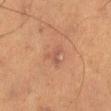Impression:
Recorded during total-body skin imaging; not selected for excision or biopsy.
Image and clinical context:
Imaged with cross-polarized lighting. The lesion is located on the leg. Longest diameter approximately 3 mm. Automated image analysis of the tile measured a border-irregularity index near 5/10 and a within-lesion color-variation index near 2.5/10. It also reported an automated nevus-likeness rating near 0 out of 100 and a lesion-detection confidence of about 100/100. A region of skin cropped from a whole-body photographic capture, roughly 15 mm wide. A male subject, aged 73–77.